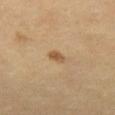Case summary:
• biopsy status: no biopsy performed (imaged during a skin exam)
• diameter: ≈2 mm
• location: the left thigh
• subject: female, approximately 70 years of age
• acquisition: total-body-photography crop, ~15 mm field of view
• lighting: cross-polarized illumination